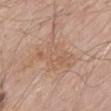Case summary:
* notes — total-body-photography surveillance lesion; no biopsy
* lesion size — about 6.5 mm
* patient — male, roughly 70 years of age
* TBP lesion metrics — an average lesion color of about L≈59 a*≈18 b*≈30 (CIELAB) and about 6 CIELAB-L* units darker than the surrounding skin
* illumination — white-light
* site — the mid back
* image source — ~15 mm tile from a whole-body skin photo